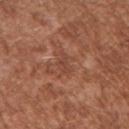{
  "biopsy_status": "not biopsied; imaged during a skin examination",
  "image": {
    "source": "total-body photography crop",
    "field_of_view_mm": 15
  },
  "lighting": "white-light",
  "patient": {
    "sex": "male",
    "age_approx": 45
  },
  "automated_metrics": {
    "area_mm2_approx": 3.5,
    "shape_asymmetry": 0.3,
    "border_irregularity_0_10": 3.0,
    "color_variation_0_10": 1.0,
    "peripheral_color_asymmetry": 0.5
  },
  "site": "right upper arm"
}